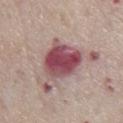Q: Was a biopsy performed?
A: total-body-photography surveillance lesion; no biopsy
Q: How was the tile lit?
A: white-light illumination
Q: Where on the body is the lesion?
A: the chest
Q: What is the imaging modality?
A: total-body-photography crop, ~15 mm field of view
Q: What are the patient's age and sex?
A: male, in their mid- to late 70s